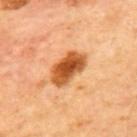{"biopsy_status": "not biopsied; imaged during a skin examination", "lighting": "cross-polarized", "automated_metrics": {"eccentricity": 0.85, "vs_skin_darker_L": 18.0, "vs_skin_contrast_norm": 11.5, "border_irregularity_0_10": 2.0, "color_variation_0_10": 5.5, "peripheral_color_asymmetry": 1.5, "nevus_likeness_0_100": 95, "lesion_detection_confidence_0_100": 100}, "patient": {"sex": "male", "age_approx": 65}, "image": {"source": "total-body photography crop", "field_of_view_mm": 15}, "site": "mid back"}